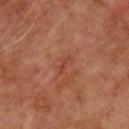Imaged during a routine full-body skin examination; the lesion was not biopsied and no histopathology is available.
Captured under cross-polarized illumination.
Automated tile analysis of the lesion measured a lesion area of about 1.5 mm². It also reported a lesion color around L≈42 a*≈29 b*≈29 in CIELAB and a normalized lesion–skin contrast near 5. And it measured a border-irregularity index near 7/10, a within-lesion color-variation index near 0/10, and a peripheral color-asymmetry measure near 0.
A male subject about 65 years old.
Approximately 2.5 mm at its widest.
Cropped from a total-body skin-imaging series; the visible field is about 15 mm.